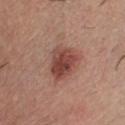- notes — no biopsy performed (imaged during a skin exam)
- image-analysis metrics — an eccentricity of roughly 0.65 and two-axis asymmetry of about 0.2
- image — total-body-photography crop, ~15 mm field of view
- tile lighting — white-light illumination
- patient — male, about 40 years old
- body site — the chest